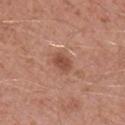notes — no biopsy performed (imaged during a skin exam)
tile lighting — white-light illumination
body site — the left lower leg
subject — male, about 60 years old
image — ~15 mm crop, total-body skin-cancer survey
lesion size — ≈2.5 mm